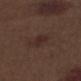Part of a total-body skin-imaging series; this lesion was reviewed on a skin check and was not flagged for biopsy.
A roughly 15 mm field-of-view crop from a total-body skin photograph.
The lesion is on the right thigh.
The total-body-photography lesion software estimated an area of roughly 5 mm², an outline eccentricity of about 0.8 (0 = round, 1 = elongated), and a symmetry-axis asymmetry near 0.25. The analysis additionally found a mean CIELAB color near L≈27 a*≈16 b*≈18 and a lesion–skin lightness drop of about 5. The software also gave border irregularity of about 2.5 on a 0–10 scale, a within-lesion color-variation index near 1.5/10, and peripheral color asymmetry of about 0.5. The analysis additionally found an automated nevus-likeness rating near 35 out of 100 and lesion-presence confidence of about 100/100.
Longest diameter approximately 3 mm.
The tile uses white-light illumination.
A male subject, about 70 years old.